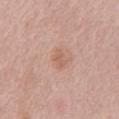| feature | finding |
|---|---|
| biopsy status | total-body-photography surveillance lesion; no biopsy |
| illumination | white-light illumination |
| subject | female, aged approximately 65 |
| site | the chest |
| image source | ~15 mm crop, total-body skin-cancer survey |
| lesion diameter | ~3 mm (longest diameter) |